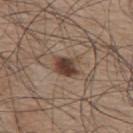follow-up=no biopsy performed (imaged during a skin exam)